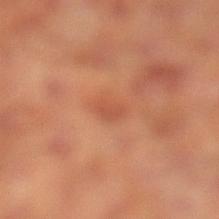Captured during whole-body skin photography for melanoma surveillance; the lesion was not biopsied. This is a cross-polarized tile. A male patient aged 63–67. About 3 mm across. The lesion is on the leg. A 15 mm close-up extracted from a 3D total-body photography capture.